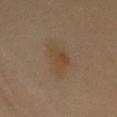Assessment: The lesion was tiled from a total-body skin photograph and was not biopsied. Context: The lesion is on the mid back. Automated tile analysis of the lesion measured a lesion color around L≈38 a*≈12 b*≈25 in CIELAB, a lesion–skin lightness drop of about 4, and a normalized lesion–skin contrast near 5. A 15 mm close-up tile from a total-body photography series done for melanoma screening. The tile uses cross-polarized illumination. Approximately 5.5 mm at its widest. The patient is a female aged 58–62.